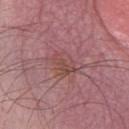Case summary:
* biopsy status — catalogued during a skin exam; not biopsied
* subject — male, about 60 years old
* image — ~15 mm crop, total-body skin-cancer survey
* body site — the chest
* tile lighting — white-light